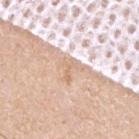Assessment:
No biopsy was performed on this lesion — it was imaged during a full skin examination and was not determined to be concerning.
Image and clinical context:
This image is a 15 mm lesion crop taken from a total-body photograph. Automated image analysis of the tile measured an area of roughly 5 mm², an outline eccentricity of about 0.45 (0 = round, 1 = elongated), and two-axis asymmetry of about 0.55. It also reported an average lesion color of about L≈71 a*≈17 b*≈31 (CIELAB), roughly 8 lightness units darker than nearby skin, and a normalized border contrast of about 6. The analysis additionally found a classifier nevus-likeness of about 0/100 and a detector confidence of about 55 out of 100 that the crop contains a lesion. Approximately 3 mm at its widest. From the upper back. This is a white-light tile. The patient is a male aged 28–32.